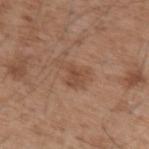Imaged during a routine full-body skin examination; the lesion was not biopsied and no histopathology is available. Measured at roughly 4 mm in maximum diameter. A male subject, aged approximately 55. On the left upper arm. Captured under white-light illumination. A region of skin cropped from a whole-body photographic capture, roughly 15 mm wide.